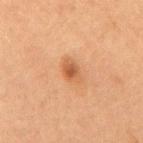follow-up: total-body-photography surveillance lesion; no biopsy | automated lesion analysis: a footprint of about 4.5 mm², a shape eccentricity near 0.75, and a shape-asymmetry score of about 0.25 (0 = symmetric); an automated nevus-likeness rating near 80 out of 100 | patient: female, in their 60s | lesion size: ~3 mm (longest diameter) | imaging modality: total-body-photography crop, ~15 mm field of view | anatomic site: the mid back.